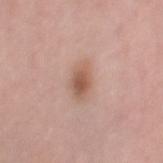biopsy status: no biopsy performed (imaged during a skin exam) | location: the right thigh | diameter: ≈3.5 mm | subject: female, approximately 50 years of age | image: total-body-photography crop, ~15 mm field of view | tile lighting: white-light | image-analysis metrics: internal color variation of about 4 on a 0–10 scale and radial color variation of about 1.5; an automated nevus-likeness rating near 95 out of 100 and a detector confidence of about 100 out of 100 that the crop contains a lesion.